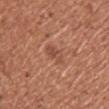<tbp_lesion>
<biopsy_status>not biopsied; imaged during a skin examination</biopsy_status>
<image>
  <source>total-body photography crop</source>
  <field_of_view_mm>15</field_of_view_mm>
</image>
<lesion_size>
  <long_diameter_mm_approx>3.0</long_diameter_mm_approx>
</lesion_size>
<patient>
  <sex>female</sex>
  <age_approx>50</age_approx>
</patient>
<lighting>white-light</lighting>
<site>chest</site>
<automated_metrics>
  <cielab_L>49</cielab_L>
  <cielab_a>25</cielab_a>
  <cielab_b>31</cielab_b>
  <vs_skin_contrast_norm>5.5</vs_skin_contrast_norm>
  <border_irregularity_0_10>3.0</border_irregularity_0_10>
  <color_variation_0_10>3.5</color_variation_0_10>
  <peripheral_color_asymmetry>1.0</peripheral_color_asymmetry>
</automated_metrics>
</tbp_lesion>